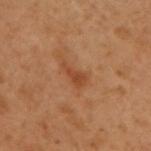The lesion was tiled from a total-body skin photograph and was not biopsied. Captured under cross-polarized illumination. A male patient aged 48–52. A 15 mm crop from a total-body photograph taken for skin-cancer surveillance. The lesion is located on the right upper arm.